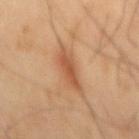Assessment: Recorded during total-body skin imaging; not selected for excision or biopsy. Image and clinical context: Longest diameter approximately 5.5 mm. Cropped from a whole-body photographic skin survey; the tile spans about 15 mm. The lesion-visualizer software estimated a lesion area of about 8.5 mm², a shape eccentricity near 0.9, and a symmetry-axis asymmetry near 0.3. The software also gave lesion-presence confidence of about 100/100. Captured under cross-polarized illumination. On the back. A male subject, approximately 45 years of age.